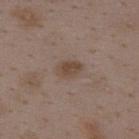| key | value |
|---|---|
| body site | the back |
| subject | female, in their mid- to late 30s |
| acquisition | 15 mm crop, total-body photography |
| automated lesion analysis | a mean CIELAB color near L≈45 a*≈14 b*≈25 and a normalized lesion–skin contrast near 7; a nevus-likeness score of about 40/100 |
| tile lighting | white-light |